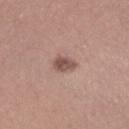From the arm.
The lesion's longest dimension is about 2.5 mm.
This is a white-light tile.
A region of skin cropped from a whole-body photographic capture, roughly 15 mm wide.
A male subject, aged around 40.